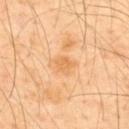Impression:
Imaged during a routine full-body skin examination; the lesion was not biopsied and no histopathology is available.
Clinical summary:
From the back. The subject is a male approximately 50 years of age. This image is a 15 mm lesion crop taken from a total-body photograph. About 2.5 mm across.